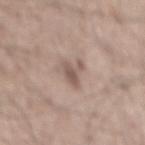This lesion was catalogued during total-body skin photography and was not selected for biopsy.
A 15 mm close-up tile from a total-body photography series done for melanoma screening.
The subject is a male aged around 55.
Imaged with white-light lighting.
Longest diameter approximately 3.5 mm.
From the mid back.
Automated image analysis of the tile measured a classifier nevus-likeness of about 5/100 and lesion-presence confidence of about 95/100.